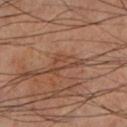notes: imaged on a skin check; not biopsied
patient: male, aged 58–62
automated metrics: a classifier nevus-likeness of about 0/100 and a lesion-detection confidence of about 90/100
acquisition: total-body-photography crop, ~15 mm field of view
location: the leg
tile lighting: cross-polarized illumination
lesion size: ~4.5 mm (longest diameter)